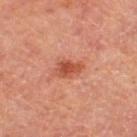Q: Was this lesion biopsied?
A: total-body-photography surveillance lesion; no biopsy
Q: Lesion size?
A: ~3 mm (longest diameter)
Q: Where on the body is the lesion?
A: the right thigh
Q: Illumination type?
A: cross-polarized
Q: Patient demographics?
A: female
Q: What is the imaging modality?
A: ~15 mm crop, total-body skin-cancer survey
Q: Automated lesion metrics?
A: a border-irregularity rating of about 2.5/10, a within-lesion color-variation index near 3.5/10, and a peripheral color-asymmetry measure near 1